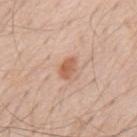The lesion was photographed on a routine skin check and not biopsied; there is no pathology result.
Longest diameter approximately 2.5 mm.
A male subject, roughly 60 years of age.
This is a white-light tile.
From the mid back.
Automated tile analysis of the lesion measured a lesion-to-skin contrast of about 7.5 (normalized; higher = more distinct). It also reported a nevus-likeness score of about 85/100 and a lesion-detection confidence of about 100/100.
A 15 mm close-up extracted from a 3D total-body photography capture.